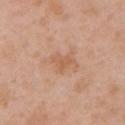| field | value |
|---|---|
| biopsy status | catalogued during a skin exam; not biopsied |
| automated metrics | a nevus-likeness score of about 0/100 and a lesion-detection confidence of about 100/100 |
| diameter | ≈2.5 mm |
| lighting | white-light illumination |
| image source | ~15 mm crop, total-body skin-cancer survey |
| site | the left upper arm |
| subject | female, roughly 40 years of age |